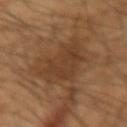follow-up: no biopsy performed (imaged during a skin exam)
image: total-body-photography crop, ~15 mm field of view
lighting: cross-polarized
location: the right forearm
subject: male, roughly 65 years of age
lesion size: about 6 mm